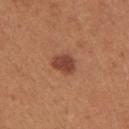Imaged during a routine full-body skin examination; the lesion was not biopsied and no histopathology is available.
Imaged with white-light lighting.
On the arm.
A 15 mm crop from a total-body photograph taken for skin-cancer surveillance.
The patient is a female roughly 30 years of age.